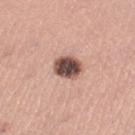Assessment:
Imaged during a routine full-body skin examination; the lesion was not biopsied and no histopathology is available.
Background:
Located on the left thigh. A female patient aged around 30. A roughly 15 mm field-of-view crop from a total-body skin photograph. Imaged with white-light lighting.